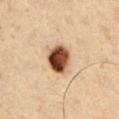• workup: total-body-photography surveillance lesion; no biopsy
• anatomic site: the left upper arm
• patient: male, about 50 years old
• lesion size: about 3.5 mm
• illumination: cross-polarized
• imaging modality: ~15 mm tile from a whole-body skin photo